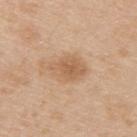Captured during whole-body skin photography for melanoma surveillance; the lesion was not biopsied.
This is a white-light tile.
Approximately 4.5 mm at its widest.
Located on the upper back.
A 15 mm close-up tile from a total-body photography series done for melanoma screening.
The subject is a male aged 33–37.